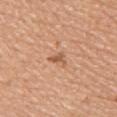Notes:
– workup: no biopsy performed (imaged during a skin exam)
– lesion size: ≈3 mm
– automated lesion analysis: an area of roughly 3 mm² and a shape eccentricity near 0.8; border irregularity of about 5 on a 0–10 scale and a peripheral color-asymmetry measure near 1
– imaging modality: ~15 mm tile from a whole-body skin photo
– lighting: white-light
– subject: female, roughly 40 years of age
– body site: the chest